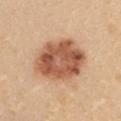Clinical impression:
Captured during whole-body skin photography for melanoma surveillance; the lesion was not biopsied.
Background:
A female subject, roughly 25 years of age. Located on the upper back. Cropped from a whole-body photographic skin survey; the tile spans about 15 mm. The lesion's longest dimension is about 6.5 mm. Automated tile analysis of the lesion measured a shape eccentricity near 0.7 and a symmetry-axis asymmetry near 0.1. The analysis additionally found a mean CIELAB color near L≈56 a*≈24 b*≈33 and a lesion-to-skin contrast of about 10.5 (normalized; higher = more distinct). The analysis additionally found border irregularity of about 1 on a 0–10 scale, internal color variation of about 6.5 on a 0–10 scale, and peripheral color asymmetry of about 2.5. It also reported an automated nevus-likeness rating near 95 out of 100 and a detector confidence of about 100 out of 100 that the crop contains a lesion.